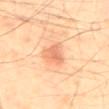biopsy status: total-body-photography surveillance lesion; no biopsy
size: ~3 mm (longest diameter)
illumination: cross-polarized
image source: total-body-photography crop, ~15 mm field of view
automated lesion analysis: a footprint of about 5.5 mm², a shape eccentricity near 0.65, and a symmetry-axis asymmetry near 0.35; an automated nevus-likeness rating near 70 out of 100 and a detector confidence of about 100 out of 100 that the crop contains a lesion
anatomic site: the mid back
patient: male, roughly 40 years of age